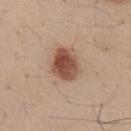<tbp_lesion>
  <image>
    <source>total-body photography crop</source>
    <field_of_view_mm>15</field_of_view_mm>
  </image>
  <site>abdomen</site>
  <lesion_size>
    <long_diameter_mm_approx>3.5</long_diameter_mm_approx>
  </lesion_size>
  <lighting>white-light</lighting>
  <patient>
    <sex>male</sex>
    <age_approx>35</age_approx>
  </patient>
</tbp_lesion>